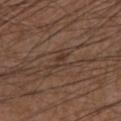notes = no biopsy performed (imaged during a skin exam); subject = male, aged around 50; imaging modality = ~15 mm crop, total-body skin-cancer survey; tile lighting = white-light; location = the arm; lesion size = about 3 mm.